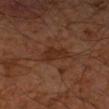Notes:
* notes · imaged on a skin check; not biopsied
* image-analysis metrics · a footprint of about 6 mm², an outline eccentricity of about 0.85 (0 = round, 1 = elongated), and a shape-asymmetry score of about 0.3 (0 = symmetric); a lesion color around L≈26 a*≈19 b*≈26 in CIELAB; border irregularity of about 3 on a 0–10 scale, a color-variation rating of about 2/10, and radial color variation of about 0.5; a classifier nevus-likeness of about 0/100 and a lesion-detection confidence of about 100/100
* lesion size · ~3.5 mm (longest diameter)
* tile lighting · cross-polarized
* imaging modality · ~15 mm tile from a whole-body skin photo
* body site · the right forearm
* subject · male, aged approximately 60